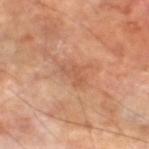<case>
<patient>
  <sex>male</sex>
  <age_approx>70</age_approx>
</patient>
<site>left lower leg</site>
<image>
  <source>total-body photography crop</source>
  <field_of_view_mm>15</field_of_view_mm>
</image>
<lesion_size>
  <long_diameter_mm_approx>3.0</long_diameter_mm_approx>
</lesion_size>
<lighting>cross-polarized</lighting>
<automated_metrics>
  <border_irregularity_0_10>6.0</border_irregularity_0_10>
  <color_variation_0_10>0.5</color_variation_0_10>
  <peripheral_color_asymmetry>0.0</peripheral_color_asymmetry>
</automated_metrics>
</case>